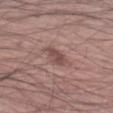- notes: no biopsy performed (imaged during a skin exam)
- lighting: white-light
- anatomic site: the left forearm
- subject: male, in their 70s
- image: 15 mm crop, total-body photography
- size: ~3.5 mm (longest diameter)